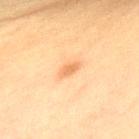Q: Was this lesion biopsied?
A: catalogued during a skin exam; not biopsied
Q: What did automated image analysis measure?
A: an area of roughly 3 mm², an eccentricity of roughly 0.9, and a symmetry-axis asymmetry near 0.25; an average lesion color of about L≈55 a*≈19 b*≈35 (CIELAB), a lesion–skin lightness drop of about 8, and a normalized border contrast of about 6; internal color variation of about 0.5 on a 0–10 scale and a peripheral color-asymmetry measure near 0
Q: How large is the lesion?
A: ~3 mm (longest diameter)
Q: What is the imaging modality?
A: ~15 mm crop, total-body skin-cancer survey
Q: Where on the body is the lesion?
A: the upper back
Q: Patient demographics?
A: male, aged approximately 60
Q: How was the tile lit?
A: cross-polarized illumination